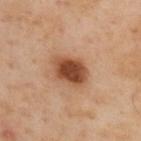Q: Is there a histopathology result?
A: catalogued during a skin exam; not biopsied
Q: Where on the body is the lesion?
A: the upper back
Q: Who is the patient?
A: male, aged 53–57
Q: Automated lesion metrics?
A: a lesion color around L≈47 a*≈24 b*≈34 in CIELAB and a normalized lesion–skin contrast near 11.5; border irregularity of about 2 on a 0–10 scale and a color-variation rating of about 5/10; a detector confidence of about 100 out of 100 that the crop contains a lesion
Q: What is the imaging modality?
A: total-body-photography crop, ~15 mm field of view
Q: Illumination type?
A: cross-polarized illumination